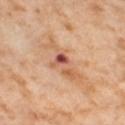Clinical impression: The lesion was tiled from a total-body skin photograph and was not biopsied. Background: On the left thigh. Imaged with cross-polarized lighting. The recorded lesion diameter is about 7 mm. A 15 mm close-up tile from a total-body photography series done for melanoma screening. The subject is a female aged around 55. An algorithmic analysis of the crop reported an automated nevus-likeness rating near 0 out of 100.